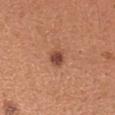Q: Was a biopsy performed?
A: total-body-photography surveillance lesion; no biopsy
Q: Where on the body is the lesion?
A: the right forearm
Q: How large is the lesion?
A: ≈2.5 mm
Q: Illumination type?
A: white-light
Q: What are the patient's age and sex?
A: female, in their 40s
Q: What is the imaging modality?
A: ~15 mm crop, total-body skin-cancer survey